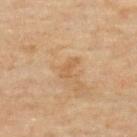<lesion>
<biopsy_status>not biopsied; imaged during a skin examination</biopsy_status>
<lighting>cross-polarized</lighting>
<site>upper back</site>
<patient>
  <sex>male</sex>
  <age_approx>45</age_approx>
</patient>
<image>
  <source>total-body photography crop</source>
  <field_of_view_mm>15</field_of_view_mm>
</image>
</lesion>